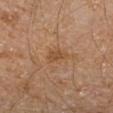Findings:
• subject: male, aged around 65
• diameter: ≈2.5 mm
• body site: the arm
• acquisition: total-body-photography crop, ~15 mm field of view
• lighting: cross-polarized
• TBP lesion metrics: a mean CIELAB color near L≈48 a*≈21 b*≈35, a lesion–skin lightness drop of about 7, and a lesion-to-skin contrast of about 6 (normalized; higher = more distinct)